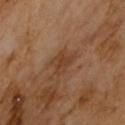Q: What kind of image is this?
A: 15 mm crop, total-body photography
Q: What did automated image analysis measure?
A: a lesion color around L≈38 a*≈20 b*≈31 in CIELAB, about 7 CIELAB-L* units darker than the surrounding skin, and a normalized lesion–skin contrast near 6.5; an automated nevus-likeness rating near 0 out of 100 and a detector confidence of about 100 out of 100 that the crop contains a lesion
Q: Patient demographics?
A: male, aged 63–67
Q: How was the tile lit?
A: cross-polarized